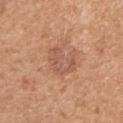This lesion was catalogued during total-body skin photography and was not selected for biopsy. From the arm. This is a white-light tile. Automated tile analysis of the lesion measured an outline eccentricity of about 0.75 (0 = round, 1 = elongated). It also reported an average lesion color of about L≈56 a*≈23 b*≈31 (CIELAB). The analysis additionally found a within-lesion color-variation index near 2.5/10 and radial color variation of about 1. About 4 mm across. A female patient aged 53 to 57. A roughly 15 mm field-of-view crop from a total-body skin photograph.